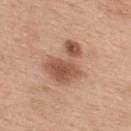Clinical impression: This lesion was catalogued during total-body skin photography and was not selected for biopsy. Acquisition and patient details: Located on the upper back. The lesion's longest dimension is about 5 mm. Automated tile analysis of the lesion measured an area of roughly 15 mm² and two-axis asymmetry of about 0.5. A region of skin cropped from a whole-body photographic capture, roughly 15 mm wide. Captured under white-light illumination. The patient is a female aged approximately 40.